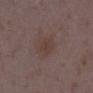biopsy_status: not biopsied; imaged during a skin examination
lighting: white-light
automated_metrics:
  area_mm2_approx: 5.5
  shape_asymmetry: 0.15
  cielab_L: 38
  cielab_a: 15
  cielab_b: 20
  vs_skin_darker_L: 5.0
  vs_skin_contrast_norm: 5.5
  border_irregularity_0_10: 1.5
  color_variation_0_10: 1.5
  peripheral_color_asymmetry: 0.5
  nevus_likeness_0_100: 0
  lesion_detection_confidence_0_100: 100
lesion_size:
  long_diameter_mm_approx: 3.0
site: leg
image:
  source: total-body photography crop
  field_of_view_mm: 15
patient:
  sex: female
  age_approx: 35